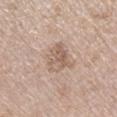Captured during whole-body skin photography for melanoma surveillance; the lesion was not biopsied.
The lesion is on the right lower leg.
A female subject aged 68 to 72.
Imaged with white-light lighting.
About 4 mm across.
Cropped from a total-body skin-imaging series; the visible field is about 15 mm.
The total-body-photography lesion software estimated an average lesion color of about L≈60 a*≈16 b*≈27 (CIELAB) and a lesion-to-skin contrast of about 6 (normalized; higher = more distinct). The software also gave an automated nevus-likeness rating near 0 out of 100 and lesion-presence confidence of about 100/100.